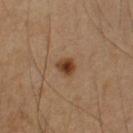Case summary:
- workup: catalogued during a skin exam; not biopsied
- TBP lesion metrics: two-axis asymmetry of about 0.25; an average lesion color of about L≈39 a*≈20 b*≈31 (CIELAB), a lesion–skin lightness drop of about 12, and a normalized lesion–skin contrast near 10.5; a border-irregularity rating of about 2/10, internal color variation of about 5 on a 0–10 scale, and peripheral color asymmetry of about 1.5; an automated nevus-likeness rating near 95 out of 100 and lesion-presence confidence of about 100/100
- body site: the left upper arm
- subject: male, in their mid- to late 60s
- illumination: cross-polarized
- diameter: ~2.5 mm (longest diameter)
- image source: ~15 mm tile from a whole-body skin photo